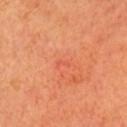Findings:
– subject: female, about 55 years old
– acquisition: 15 mm crop, total-body photography
– size: ~1.5 mm (longest diameter)
– body site: the head or neck